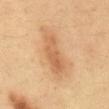The lesion was tiled from a total-body skin photograph and was not biopsied. The recorded lesion diameter is about 6.5 mm. A male subject aged approximately 35. A close-up tile cropped from a whole-body skin photograph, about 15 mm across. This is a cross-polarized tile. The lesion is on the mid back.